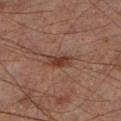notes — catalogued during a skin exam; not biopsied | patient — male, aged approximately 65 | anatomic site — the left lower leg | acquisition — 15 mm crop, total-body photography.